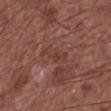workup: catalogued during a skin exam; not biopsied | acquisition: ~15 mm crop, total-body skin-cancer survey | body site: the left lower leg | lesion size: ≈4 mm | tile lighting: white-light illumination | subject: male, about 65 years old.